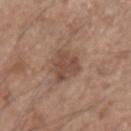Clinical impression:
The lesion was tiled from a total-body skin photograph and was not biopsied.
Clinical summary:
Automated tile analysis of the lesion measured a within-lesion color-variation index near 3/10. From the left forearm. About 4.5 mm across. A male patient, approximately 55 years of age. Cropped from a total-body skin-imaging series; the visible field is about 15 mm. Captured under white-light illumination.